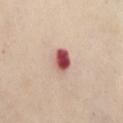Assessment:
Part of a total-body skin-imaging series; this lesion was reviewed on a skin check and was not flagged for biopsy.
Context:
Approximately 3 mm at its widest. A roughly 15 mm field-of-view crop from a total-body skin photograph. Located on the abdomen. Imaged with cross-polarized lighting. A female subject aged 53 to 57.